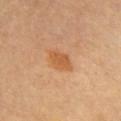The lesion was tiled from a total-body skin photograph and was not biopsied.
The recorded lesion diameter is about 3.5 mm.
A roughly 15 mm field-of-view crop from a total-body skin photograph.
This is a cross-polarized tile.
Automated image analysis of the tile measured a footprint of about 6.5 mm² and an outline eccentricity of about 0.75 (0 = round, 1 = elongated). The software also gave a within-lesion color-variation index near 2/10 and peripheral color asymmetry of about 0.5. And it measured a classifier nevus-likeness of about 70/100.
A patient about 60 years old.
Located on the chest.